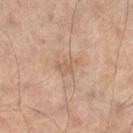| feature | finding |
|---|---|
| follow-up | imaged on a skin check; not biopsied |
| patient | male, aged 48 to 52 |
| imaging modality | ~15 mm tile from a whole-body skin photo |
| site | the left leg |